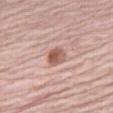Clinical impression: The lesion was photographed on a routine skin check and not biopsied; there is no pathology result. Acquisition and patient details: The tile uses white-light illumination. The subject is a male about 80 years old. The lesion is located on the leg. The lesion-visualizer software estimated an average lesion color of about L≈57 a*≈22 b*≈25 (CIELAB) and a lesion-to-skin contrast of about 8.5 (normalized; higher = more distinct). The analysis additionally found a border-irregularity index near 1/10, a within-lesion color-variation index near 6/10, and a peripheral color-asymmetry measure near 2. The analysis additionally found a nevus-likeness score of about 95/100. This image is a 15 mm lesion crop taken from a total-body photograph.